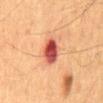The lesion was photographed on a routine skin check and not biopsied; there is no pathology result. On the mid back. A 15 mm close-up tile from a total-body photography series done for melanoma screening. A male patient in their mid- to late 60s. Captured under cross-polarized illumination. Measured at roughly 3.5 mm in maximum diameter.